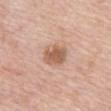The lesion was photographed on a routine skin check and not biopsied; there is no pathology result. The lesion is on the abdomen. Approximately 3.5 mm at its widest. A 15 mm close-up extracted from a 3D total-body photography capture. A female subject aged approximately 75. An algorithmic analysis of the crop reported a mean CIELAB color near L≈59 a*≈20 b*≈31, a lesion–skin lightness drop of about 11, and a lesion-to-skin contrast of about 7.5 (normalized; higher = more distinct). The software also gave border irregularity of about 1.5 on a 0–10 scale, internal color variation of about 3 on a 0–10 scale, and a peripheral color-asymmetry measure near 1.